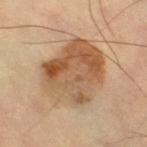Q: Was a biopsy performed?
A: total-body-photography surveillance lesion; no biopsy
Q: Lesion location?
A: the right thigh
Q: Lesion size?
A: ~7.5 mm (longest diameter)
Q: Who is the patient?
A: male, approximately 60 years of age
Q: How was the tile lit?
A: cross-polarized
Q: What kind of image is this?
A: 15 mm crop, total-body photography
Q: Automated lesion metrics?
A: border irregularity of about 2 on a 0–10 scale and radial color variation of about 2.5